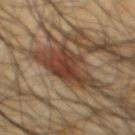Q: Is there a histopathology result?
A: total-body-photography surveillance lesion; no biopsy
Q: Where on the body is the lesion?
A: the mid back
Q: What did automated image analysis measure?
A: a lesion area of about 19 mm², an outline eccentricity of about 0.85 (0 = round, 1 = elongated), and two-axis asymmetry of about 0.4; an average lesion color of about L≈38 a*≈17 b*≈26 (CIELAB) and a lesion-to-skin contrast of about 10 (normalized; higher = more distinct); a border-irregularity index near 5.5/10, internal color variation of about 5.5 on a 0–10 scale, and peripheral color asymmetry of about 2; an automated nevus-likeness rating near 100 out of 100 and a lesion-detection confidence of about 90/100
Q: Patient demographics?
A: male, aged 63 to 67
Q: How large is the lesion?
A: about 7 mm
Q: What kind of image is this?
A: 15 mm crop, total-body photography
Q: Illumination type?
A: cross-polarized